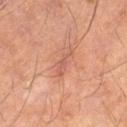Clinical impression:
This lesion was catalogued during total-body skin photography and was not selected for biopsy.